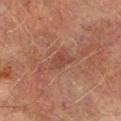Imaged during a routine full-body skin examination; the lesion was not biopsied and no histopathology is available. An algorithmic analysis of the crop reported internal color variation of about 2 on a 0–10 scale and peripheral color asymmetry of about 0.5. The analysis additionally found an automated nevus-likeness rating near 0 out of 100 and a detector confidence of about 100 out of 100 that the crop contains a lesion. The lesion's longest dimension is about 3.5 mm. Imaged with cross-polarized lighting. Located on the left lower leg. The subject is a male approximately 75 years of age. A lesion tile, about 15 mm wide, cut from a 3D total-body photograph.